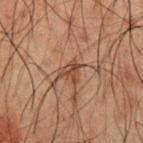Q: Where on the body is the lesion?
A: the chest
Q: Who is the patient?
A: male, approximately 50 years of age
Q: What is the imaging modality?
A: ~15 mm tile from a whole-body skin photo